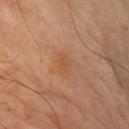Case summary:
• notes · no biopsy performed (imaged during a skin exam)
• image source · total-body-photography crop, ~15 mm field of view
• location · the arm
• subject · male, aged 63–67